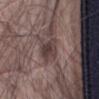Clinical impression:
Captured during whole-body skin photography for melanoma surveillance; the lesion was not biopsied.
Image and clinical context:
Approximately 3 mm at its widest. A male subject aged 78–82. A 15 mm close-up tile from a total-body photography series done for melanoma screening. On the front of the torso. Imaged with white-light lighting.